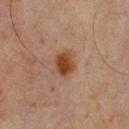This is a cross-polarized tile.
The lesion is on the upper back.
A male patient, aged around 65.
The total-body-photography lesion software estimated an eccentricity of roughly 0.65 and two-axis asymmetry of about 0.2. It also reported border irregularity of about 2 on a 0–10 scale, a color-variation rating of about 3/10, and a peripheral color-asymmetry measure near 1.
This image is a 15 mm lesion crop taken from a total-body photograph.
The lesion's longest dimension is about 3 mm.